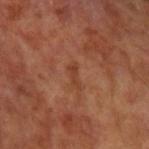This lesion was catalogued during total-body skin photography and was not selected for biopsy. The subject is a male aged 63 to 67. Longest diameter approximately 3 mm. A 15 mm close-up tile from a total-body photography series done for melanoma screening. The lesion is on the left upper arm. The lesion-visualizer software estimated a footprint of about 2.5 mm² and two-axis asymmetry of about 0.45.